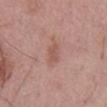biopsy_status: not biopsied; imaged during a skin examination
lighting: white-light
site: back
lesion_size:
  long_diameter_mm_approx: 3.0
patient:
  sex: male
  age_approx: 55
image:
  source: total-body photography crop
  field_of_view_mm: 15